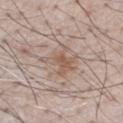Q: Was this lesion biopsied?
A: total-body-photography surveillance lesion; no biopsy
Q: Automated lesion metrics?
A: a lesion area of about 8.5 mm² and a shape-asymmetry score of about 0.45 (0 = symmetric); a classifier nevus-likeness of about 10/100
Q: Who is the patient?
A: male, in their mid- to late 50s
Q: What kind of image is this?
A: ~15 mm tile from a whole-body skin photo
Q: Illumination type?
A: white-light
Q: What is the lesion's diameter?
A: ~4 mm (longest diameter)